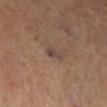No biopsy was performed on this lesion — it was imaged during a full skin examination and was not determined to be concerning. A lesion tile, about 15 mm wide, cut from a 3D total-body photograph. Longest diameter approximately 3 mm. The lesion is located on the right lower leg. A female subject in their 70s. The lesion-visualizer software estimated a detector confidence of about 80 out of 100 that the crop contains a lesion. The tile uses cross-polarized illumination.